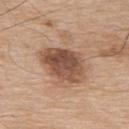  biopsy_status: not biopsied; imaged during a skin examination
  image:
    source: total-body photography crop
    field_of_view_mm: 15
  site: upper back
  patient:
    sex: male
    age_approx: 75